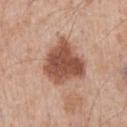A 15 mm crop from a total-body photograph taken for skin-cancer surveillance. A male patient aged 53–57. The lesion is on the front of the torso.